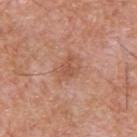<tbp_lesion>
  <biopsy_status>not biopsied; imaged during a skin examination</biopsy_status>
  <automated_metrics>
    <cielab_L>55</cielab_L>
    <cielab_a>23</cielab_a>
    <cielab_b>31</cielab_b>
    <vs_skin_contrast_norm>5.5</vs_skin_contrast_norm>
    <border_irregularity_0_10>2.5</border_irregularity_0_10>
    <color_variation_0_10>3.5</color_variation_0_10>
    <peripheral_color_asymmetry>1.0</peripheral_color_asymmetry>
  </automated_metrics>
  <lighting>white-light</lighting>
  <image>
    <source>total-body photography crop</source>
    <field_of_view_mm>15</field_of_view_mm>
  </image>
  <site>upper back</site>
  <patient>
    <sex>male</sex>
    <age_approx>55</age_approx>
  </patient>
  <lesion_size>
    <long_diameter_mm_approx>3.5</long_diameter_mm_approx>
  </lesion_size>
</tbp_lesion>